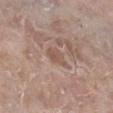No biopsy was performed on this lesion — it was imaged during a full skin examination and was not determined to be concerning. On the right lower leg. A female subject aged around 75. Measured at roughly 3.5 mm in maximum diameter. The tile uses white-light illumination. A 15 mm crop from a total-body photograph taken for skin-cancer surveillance.